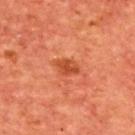patient = male, in their mid-60s
location = the back
image-analysis metrics = a lesion area of about 4 mm², an eccentricity of roughly 0.8, and a symmetry-axis asymmetry near 0.3; a border-irregularity index near 2.5/10 and a color-variation rating of about 2/10; an automated nevus-likeness rating near 45 out of 100
image = 15 mm crop, total-body photography
size = ~3 mm (longest diameter)